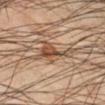Part of a total-body skin-imaging series; this lesion was reviewed on a skin check and was not flagged for biopsy. Imaged with cross-polarized lighting. The subject is a male aged 48–52. The lesion is located on the right lower leg. A close-up tile cropped from a whole-body skin photograph, about 15 mm across. The lesion-visualizer software estimated a classifier nevus-likeness of about 30/100 and a detector confidence of about 80 out of 100 that the crop contains a lesion. Longest diameter approximately 6 mm.